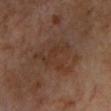Q: Was a biopsy performed?
A: imaged on a skin check; not biopsied
Q: Patient demographics?
A: aged around 65
Q: Lesion size?
A: ≈6 mm
Q: What is the imaging modality?
A: ~15 mm tile from a whole-body skin photo
Q: Illumination type?
A: cross-polarized
Q: Lesion location?
A: the left upper arm